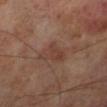Case summary:
– follow-up: total-body-photography surveillance lesion; no biopsy
– subject: male, roughly 70 years of age
– image-analysis metrics: a lesion color around L≈39 a*≈19 b*≈25 in CIELAB; a border-irregularity rating of about 4/10 and a color-variation rating of about 2.5/10; a nevus-likeness score of about 0/100
– body site: the leg
– acquisition: total-body-photography crop, ~15 mm field of view